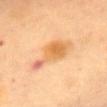Context:
Longest diameter approximately 7 mm. A 15 mm close-up extracted from a 3D total-body photography capture. Located on the chest. A female subject, aged around 60. Captured under cross-polarized illumination. The total-body-photography lesion software estimated a mean CIELAB color near L≈60 a*≈21 b*≈39 and about 9 CIELAB-L* units darker than the surrounding skin. The software also gave an automated nevus-likeness rating near 55 out of 100.